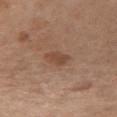Q: Was a biopsy performed?
A: total-body-photography surveillance lesion; no biopsy
Q: What are the patient's age and sex?
A: female, aged approximately 55
Q: Where on the body is the lesion?
A: the chest
Q: What lighting was used for the tile?
A: white-light
Q: Lesion size?
A: ≈3 mm
Q: What kind of image is this?
A: ~15 mm crop, total-body skin-cancer survey